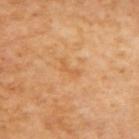Impression:
Recorded during total-body skin imaging; not selected for excision or biopsy.
Acquisition and patient details:
A 15 mm close-up extracted from a 3D total-body photography capture. The patient is a female about 55 years old. From the right upper arm.